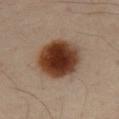Q: Who is the patient?
A: male, aged approximately 55
Q: What is the lesion's diameter?
A: ≈6 mm
Q: How was this image acquired?
A: total-body-photography crop, ~15 mm field of view
Q: Automated lesion metrics?
A: an average lesion color of about L≈37 a*≈20 b*≈29 (CIELAB) and a lesion-to-skin contrast of about 15.5 (normalized; higher = more distinct)
Q: Where on the body is the lesion?
A: the front of the torso
Q: Illumination type?
A: cross-polarized illumination